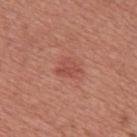Findings:
* biopsy status — imaged on a skin check; not biopsied
* patient — male, approximately 45 years of age
* lesion diameter — ~3 mm (longest diameter)
* image source — ~15 mm crop, total-body skin-cancer survey
* automated metrics — an eccentricity of roughly 0.7 and a symmetry-axis asymmetry near 0.4
* location — the chest